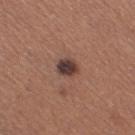This lesion was catalogued during total-body skin photography and was not selected for biopsy. From the leg. A 15 mm close-up tile from a total-body photography series done for melanoma screening. The patient is a female aged around 35.